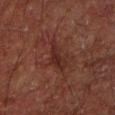Case summary:
- biopsy status · catalogued during a skin exam; not biopsied
- lighting · cross-polarized
- lesion diameter · ~4 mm (longest diameter)
- TBP lesion metrics · a lesion area of about 5.5 mm², a shape eccentricity near 0.9, and two-axis asymmetry of about 0.45; a mean CIELAB color near L≈20 a*≈18 b*≈18, about 6 CIELAB-L* units darker than the surrounding skin, and a normalized border contrast of about 7.5; a border-irregularity index near 5/10, a color-variation rating of about 3/10, and peripheral color asymmetry of about 1; an automated nevus-likeness rating near 0 out of 100 and lesion-presence confidence of about 90/100
- body site · the arm
- patient · male, aged 48 to 52
- acquisition · ~15 mm crop, total-body skin-cancer survey